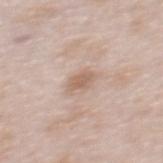workup: imaged on a skin check; not biopsied
imaging modality: total-body-photography crop, ~15 mm field of view
anatomic site: the upper back
diameter: ≈2.5 mm
patient: female, approximately 65 years of age
tile lighting: white-light